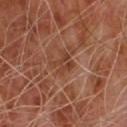workup: no biopsy performed (imaged during a skin exam)
diameter: ~2.5 mm (longest diameter)
acquisition: total-body-photography crop, ~15 mm field of view
subject: male, about 65 years old